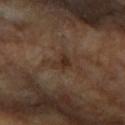The lesion was tiled from a total-body skin photograph and was not biopsied. The lesion is located on the arm. The patient is a female about 70 years old. A 15 mm crop from a total-body photograph taken for skin-cancer surveillance.